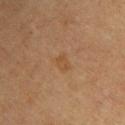biopsy status: catalogued during a skin exam; not biopsied
body site: the head or neck
automated metrics: a lesion area of about 4 mm² and a shape-asymmetry score of about 0.3 (0 = symmetric); a border-irregularity index near 3/10 and peripheral color asymmetry of about 0.5; an automated nevus-likeness rating near 5 out of 100 and a lesion-detection confidence of about 100/100
size: about 2.5 mm
image: total-body-photography crop, ~15 mm field of view
subject: female, aged 63 to 67